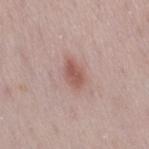<tbp_lesion>
  <biopsy_status>not biopsied; imaged during a skin examination</biopsy_status>
  <image>
    <source>total-body photography crop</source>
    <field_of_view_mm>15</field_of_view_mm>
  </image>
  <site>leg</site>
  <patient>
    <sex>female</sex>
    <age_approx>30</age_approx>
  </patient>
  <lesion_size>
    <long_diameter_mm_approx>3.5</long_diameter_mm_approx>
  </lesion_size>
  <lighting>white-light</lighting>
</tbp_lesion>